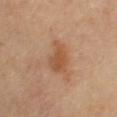Clinical impression: The lesion was photographed on a routine skin check and not biopsied; there is no pathology result. Clinical summary: The lesion is on the left upper arm. The recorded lesion diameter is about 3.5 mm. Automated tile analysis of the lesion measured border irregularity of about 3.5 on a 0–10 scale, a color-variation rating of about 3/10, and radial color variation of about 1. A 15 mm crop from a total-body photograph taken for skin-cancer surveillance.